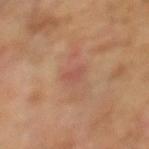Impression:
Recorded during total-body skin imaging; not selected for excision or biopsy.
Acquisition and patient details:
Approximately 2.5 mm at its widest. A male subject in their mid- to late 60s. The total-body-photography lesion software estimated a lesion color around L≈52 a*≈25 b*≈29 in CIELAB and about 6 CIELAB-L* units darker than the surrounding skin. The software also gave a border-irregularity index near 3.5/10, internal color variation of about 0.5 on a 0–10 scale, and radial color variation of about 0. And it measured a classifier nevus-likeness of about 0/100 and a detector confidence of about 100 out of 100 that the crop contains a lesion. A lesion tile, about 15 mm wide, cut from a 3D total-body photograph. Located on the left lower leg. Imaged with cross-polarized lighting.